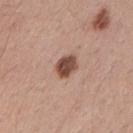Imaged during a routine full-body skin examination; the lesion was not biopsied and no histopathology is available. From the left upper arm. Automated tile analysis of the lesion measured an area of roughly 5.5 mm², an outline eccentricity of about 0.65 (0 = round, 1 = elongated), and a shape-asymmetry score of about 0.2 (0 = symmetric). The software also gave a lesion color around L≈47 a*≈21 b*≈26 in CIELAB, about 17 CIELAB-L* units darker than the surrounding skin, and a normalized border contrast of about 11.5. The analysis additionally found a border-irregularity index near 2/10, internal color variation of about 3.5 on a 0–10 scale, and radial color variation of about 1. It also reported an automated nevus-likeness rating near 80 out of 100. A male patient, aged approximately 40. A region of skin cropped from a whole-body photographic capture, roughly 15 mm wide. The recorded lesion diameter is about 3 mm.